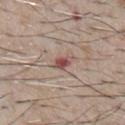The lesion was photographed on a routine skin check and not biopsied; there is no pathology result. A male subject roughly 65 years of age. From the chest. The total-body-photography lesion software estimated an eccentricity of roughly 0.8 and a symmetry-axis asymmetry near 0.2. It also reported a mean CIELAB color near L≈51 a*≈22 b*≈21 and a normalized lesion–skin contrast near 8.5. The tile uses white-light illumination. A lesion tile, about 15 mm wide, cut from a 3D total-body photograph.